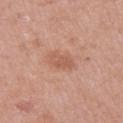biopsy status: total-body-photography surveillance lesion; no biopsy | size: ~3 mm (longest diameter) | body site: the right upper arm | imaging modality: ~15 mm tile from a whole-body skin photo | patient: female, aged around 50 | tile lighting: white-light illumination.